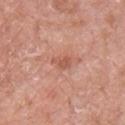Q: Lesion location?
A: the chest
Q: Who is the patient?
A: female, aged approximately 60
Q: What is the imaging modality?
A: 15 mm crop, total-body photography